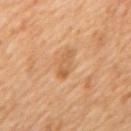A 15 mm close-up tile from a total-body photography series done for melanoma screening. A male patient, in their mid- to late 60s. The recorded lesion diameter is about 3.5 mm. This is a cross-polarized tile. The lesion is on the upper back.